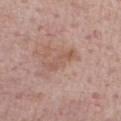Clinical impression:
Captured during whole-body skin photography for melanoma surveillance; the lesion was not biopsied.
Image and clinical context:
This is a white-light tile. A lesion tile, about 15 mm wide, cut from a 3D total-body photograph. On the chest. Automated tile analysis of the lesion measured a nevus-likeness score of about 0/100 and a lesion-detection confidence of about 100/100. The subject is a male approximately 75 years of age.